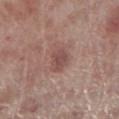Impression: The lesion was tiled from a total-body skin photograph and was not biopsied. Image and clinical context: The tile uses white-light illumination. A region of skin cropped from a whole-body photographic capture, roughly 15 mm wide. The total-body-photography lesion software estimated a footprint of about 5 mm², an outline eccentricity of about 0.7 (0 = round, 1 = elongated), and a shape-asymmetry score of about 0.25 (0 = symmetric). The software also gave an average lesion color of about L≈48 a*≈21 b*≈22 (CIELAB), about 9 CIELAB-L* units darker than the surrounding skin, and a lesion-to-skin contrast of about 7 (normalized; higher = more distinct). The software also gave a border-irregularity index near 2.5/10, a color-variation rating of about 2/10, and peripheral color asymmetry of about 0.5. The software also gave a classifier nevus-likeness of about 10/100 and a detector confidence of about 100 out of 100 that the crop contains a lesion. On the right lower leg. A male subject, aged approximately 70.